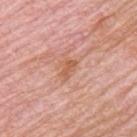biopsy_status: not biopsied; imaged during a skin examination
image:
  source: total-body photography crop
  field_of_view_mm: 15
lesion_size:
  long_diameter_mm_approx: 2.5
patient:
  sex: male
  age_approx: 65
automated_metrics:
  area_mm2_approx: 3.5
  eccentricity: 0.8
  cielab_L: 59
  cielab_a: 25
  cielab_b: 34
  vs_skin_darker_L: 8.0
  vs_skin_contrast_norm: 6.5
  border_irregularity_0_10: 3.5
  color_variation_0_10: 1.0
  peripheral_color_asymmetry: 0.0
site: upper back